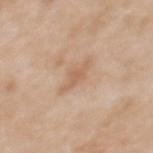| feature | finding |
|---|---|
| workup | imaged on a skin check; not biopsied |
| patient | female, roughly 40 years of age |
| anatomic site | the upper back |
| image source | total-body-photography crop, ~15 mm field of view |